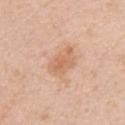  biopsy_status: not biopsied; imaged during a skin examination
  site: right upper arm
  automated_metrics:
    border_irregularity_0_10: 2.0
    color_variation_0_10: 3.0
    lesion_detection_confidence_0_100: 100
  patient:
    sex: female
    age_approx: 45
  image:
    source: total-body photography crop
    field_of_view_mm: 15
  lighting: white-light